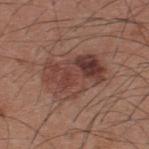Part of a total-body skin-imaging series; this lesion was reviewed on a skin check and was not flagged for biopsy. A region of skin cropped from a whole-body photographic capture, roughly 15 mm wide. The lesion-visualizer software estimated a mean CIELAB color near L≈41 a*≈21 b*≈24, roughly 10 lightness units darker than nearby skin, and a normalized lesion–skin contrast near 8. Imaged with white-light lighting. From the back. A male subject, aged 33–37.